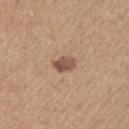The lesion was tiled from a total-body skin photograph and was not biopsied. The lesion is located on the left upper arm. A 15 mm close-up extracted from a 3D total-body photography capture. This is a white-light tile. A male subject in their mid- to late 60s.